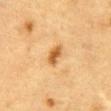  biopsy_status: not biopsied; imaged during a skin examination
  image:
    source: total-body photography crop
    field_of_view_mm: 15
  patient:
    sex: male
    age_approx: 85+
  site: abdomen